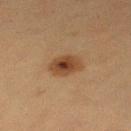No biopsy was performed on this lesion — it was imaged during a full skin examination and was not determined to be concerning.